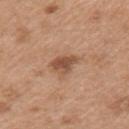| feature | finding |
|---|---|
| subject | female, about 40 years old |
| diameter | about 3 mm |
| lighting | white-light illumination |
| anatomic site | the arm |
| image source | ~15 mm crop, total-body skin-cancer survey |
| automated metrics | a nevus-likeness score of about 60/100 |